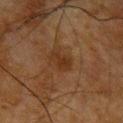Findings:
- workup — imaged on a skin check; not biopsied
- automated lesion analysis — a color-variation rating of about 2.5/10 and a peripheral color-asymmetry measure near 1; lesion-presence confidence of about 100/100
- patient — male, aged 63–67
- site — the left upper arm
- acquisition — ~15 mm tile from a whole-body skin photo
- diameter — ≈3 mm
- tile lighting — cross-polarized